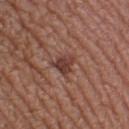Clinical impression:
Imaged during a routine full-body skin examination; the lesion was not biopsied and no histopathology is available.
Image and clinical context:
Cropped from a whole-body photographic skin survey; the tile spans about 15 mm. The lesion is on the left thigh. Automated image analysis of the tile measured an average lesion color of about L≈39 a*≈22 b*≈24 (CIELAB), roughly 10 lightness units darker than nearby skin, and a normalized lesion–skin contrast near 8.5. Longest diameter approximately 3 mm. Captured under white-light illumination. A female subject, approximately 40 years of age.